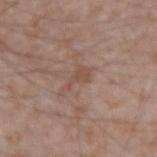The lesion was tiled from a total-body skin photograph and was not biopsied. On the right forearm. Cropped from a whole-body photographic skin survey; the tile spans about 15 mm. A male patient, in their mid- to late 70s.